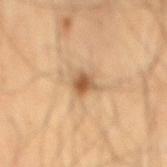Clinical impression:
This lesion was catalogued during total-body skin photography and was not selected for biopsy.
Acquisition and patient details:
The subject is a male aged approximately 60. A 15 mm close-up extracted from a 3D total-body photography capture. The recorded lesion diameter is about 2.5 mm. Imaged with cross-polarized lighting. The lesion is on the mid back.